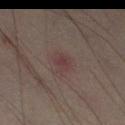Notes:
* notes — total-body-photography surveillance lesion; no biopsy
* location — the left thigh
* subject — male, aged 48 to 52
* image — 15 mm crop, total-body photography
* diameter — ~3 mm (longest diameter)
* automated lesion analysis — internal color variation of about 2 on a 0–10 scale and a peripheral color-asymmetry measure near 0.5; a classifier nevus-likeness of about 5/100 and a detector confidence of about 100 out of 100 that the crop contains a lesion